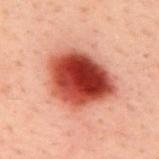The lesion was photographed on a routine skin check and not biopsied; there is no pathology result.
Cropped from a total-body skin-imaging series; the visible field is about 15 mm.
The total-body-photography lesion software estimated an automated nevus-likeness rating near 100 out of 100.
The lesion is located on the upper back.
The patient is a male about 40 years old.
Measured at roughly 7 mm in maximum diameter.